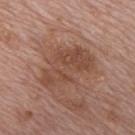The recorded lesion diameter is about 6.5 mm.
Located on the upper back.
This is a white-light tile.
A 15 mm close-up extracted from a 3D total-body photography capture.
A male patient, about 70 years old.